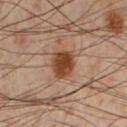This lesion was catalogued during total-body skin photography and was not selected for biopsy.
A 15 mm close-up extracted from a 3D total-body photography capture.
The lesion's longest dimension is about 3.5 mm.
The subject is a male aged 53–57.
The lesion is on the leg.
Imaged with cross-polarized lighting.